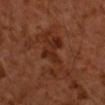Part of a total-body skin-imaging series; this lesion was reviewed on a skin check and was not flagged for biopsy.
An algorithmic analysis of the crop reported an outline eccentricity of about 0.9 (0 = round, 1 = elongated) and two-axis asymmetry of about 0.5. The software also gave a border-irregularity rating of about 8.5/10, internal color variation of about 3.5 on a 0–10 scale, and a peripheral color-asymmetry measure near 1. It also reported a classifier nevus-likeness of about 5/100.
On the right upper arm.
A male patient approximately 60 years of age.
This is a cross-polarized tile.
Cropped from a whole-body photographic skin survey; the tile spans about 15 mm.
The recorded lesion diameter is about 7 mm.